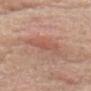Impression: The lesion was photographed on a routine skin check and not biopsied; there is no pathology result. Clinical summary: The lesion is located on the chest. The subject is a male approximately 65 years of age. A lesion tile, about 15 mm wide, cut from a 3D total-body photograph.